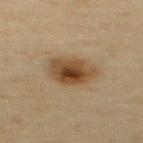| field | value |
|---|---|
| biopsy status | catalogued during a skin exam; not biopsied |
| site | the upper back |
| subject | male, about 45 years old |
| image source | ~15 mm tile from a whole-body skin photo |